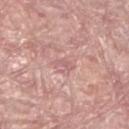Impression: The lesion was photographed on a routine skin check and not biopsied; there is no pathology result.